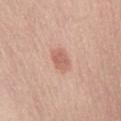Impression:
Captured during whole-body skin photography for melanoma surveillance; the lesion was not biopsied.
Acquisition and patient details:
A region of skin cropped from a whole-body photographic capture, roughly 15 mm wide. The tile uses white-light illumination. Automated image analysis of the tile measured a mean CIELAB color near L≈61 a*≈24 b*≈28, about 10 CIELAB-L* units darker than the surrounding skin, and a normalized border contrast of about 6.5. And it measured a border-irregularity rating of about 2.5/10 and a within-lesion color-variation index near 2/10. The software also gave a detector confidence of about 100 out of 100 that the crop contains a lesion. The lesion is located on the chest. The patient is a female in their mid-60s.